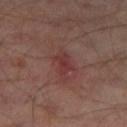notes=total-body-photography surveillance lesion; no biopsy.